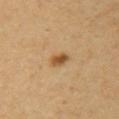image:
  source: total-body photography crop
  field_of_view_mm: 15
lesion_size:
  long_diameter_mm_approx: 2.5
site: right upper arm
patient:
  sex: female
  age_approx: 35
lighting: cross-polarized